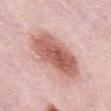Captured during whole-body skin photography for melanoma surveillance; the lesion was not biopsied. A lesion tile, about 15 mm wide, cut from a 3D total-body photograph. The lesion is on the abdomen. Measured at roughly 7 mm in maximum diameter. This is a white-light tile. A male patient, aged 53–57.